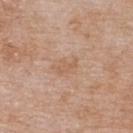Captured during whole-body skin photography for melanoma surveillance; the lesion was not biopsied.
The recorded lesion diameter is about 3 mm.
A female patient aged approximately 75.
The lesion is on the upper back.
This image is a 15 mm lesion crop taken from a total-body photograph.
Imaged with white-light lighting.